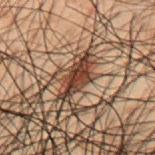workup: no biopsy performed (imaged during a skin exam) | body site: the mid back | automated lesion analysis: a lesion area of about 8.5 mm², a shape eccentricity near 0.9, and a shape-asymmetry score of about 0.3 (0 = symmetric); an automated nevus-likeness rating near 100 out of 100 and lesion-presence confidence of about 55/100 | subject: male, aged approximately 50 | diameter: about 5 mm | illumination: cross-polarized | imaging modality: ~15 mm tile from a whole-body skin photo.